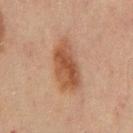Q: Is there a histopathology result?
A: catalogued during a skin exam; not biopsied
Q: What kind of image is this?
A: ~15 mm tile from a whole-body skin photo
Q: Automated lesion metrics?
A: a border-irregularity rating of about 2.5/10, a color-variation rating of about 4.5/10, and radial color variation of about 1.5
Q: What is the lesion's diameter?
A: about 6 mm
Q: Illumination type?
A: cross-polarized illumination
Q: What are the patient's age and sex?
A: male, roughly 70 years of age
Q: Lesion location?
A: the back